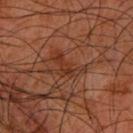Q: What lighting was used for the tile?
A: cross-polarized
Q: Lesion size?
A: ~4 mm (longest diameter)
Q: Automated lesion metrics?
A: a footprint of about 5.5 mm², a shape eccentricity near 0.85, and a shape-asymmetry score of about 0.6 (0 = symmetric); border irregularity of about 8 on a 0–10 scale and a within-lesion color-variation index near 1.5/10; an automated nevus-likeness rating near 0 out of 100 and a detector confidence of about 95 out of 100 that the crop contains a lesion
Q: What are the patient's age and sex?
A: male, about 60 years old
Q: Lesion location?
A: the left forearm
Q: How was this image acquired?
A: ~15 mm crop, total-body skin-cancer survey